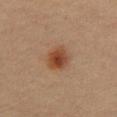Recorded during total-body skin imaging; not selected for excision or biopsy.
This is a cross-polarized tile.
The lesion is on the abdomen.
The subject is a male aged around 65.
Approximately 4 mm at its widest.
A 15 mm close-up extracted from a 3D total-body photography capture.
Automated tile analysis of the lesion measured a lesion area of about 8 mm², a shape eccentricity near 0.75, and a shape-asymmetry score of about 0.2 (0 = symmetric). The software also gave a border-irregularity index near 2/10, a color-variation rating of about 6/10, and peripheral color asymmetry of about 2.